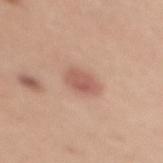{
  "biopsy_status": "not biopsied; imaged during a skin examination",
  "lighting": "white-light",
  "image": {
    "source": "total-body photography crop",
    "field_of_view_mm": 15
  },
  "patient": {
    "sex": "female",
    "age_approx": 35
  },
  "automated_metrics": {
    "area_mm2_approx": 5.0,
    "eccentricity": 0.75,
    "border_irregularity_0_10": 2.0,
    "color_variation_0_10": 3.0,
    "peripheral_color_asymmetry": 1.0
  },
  "site": "chest"
}